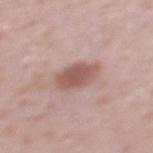workup = no biopsy performed (imaged during a skin exam); subject = female, in their 40s; body site = the back; lesion diameter = ≈4 mm; tile lighting = white-light illumination; image = 15 mm crop, total-body photography.